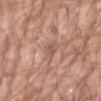Impression:
Recorded during total-body skin imaging; not selected for excision or biopsy.
Acquisition and patient details:
Captured under white-light illumination. A 15 mm crop from a total-body photograph taken for skin-cancer surveillance. From the left forearm. A male patient, aged around 60.